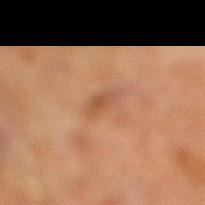| key | value |
|---|---|
| biopsy status | no biopsy performed (imaged during a skin exam) |
| patient | male, in their 70s |
| site | the right lower leg |
| image | 15 mm crop, total-body photography |
| TBP lesion metrics | an outline eccentricity of about 0.95 (0 = round, 1 = elongated) and a shape-asymmetry score of about 0.3 (0 = symmetric) |
| lighting | cross-polarized |
| lesion diameter | ≈3.5 mm |